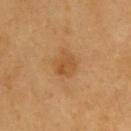biopsy status: catalogued during a skin exam; not biopsied | illumination: cross-polarized | patient: male, about 60 years old | TBP lesion metrics: a footprint of about 5.5 mm², a shape eccentricity near 0.4, and a shape-asymmetry score of about 0.25 (0 = symmetric); a mean CIELAB color near L≈51 a*≈22 b*≈40, a lesion–skin lightness drop of about 7, and a normalized border contrast of about 6; a color-variation rating of about 3.5/10; a nevus-likeness score of about 40/100 and a detector confidence of about 100 out of 100 that the crop contains a lesion | lesion diameter: about 3 mm | acquisition: total-body-photography crop, ~15 mm field of view | body site: the upper back.